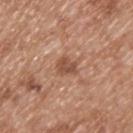notes: no biopsy performed (imaged during a skin exam)
lesion size: ≈2.5 mm
image-analysis metrics: an outline eccentricity of about 0.55 (0 = round, 1 = elongated) and a symmetry-axis asymmetry near 0.25; about 10 CIELAB-L* units darker than the surrounding skin
imaging modality: 15 mm crop, total-body photography
body site: the back
patient: male, approximately 65 years of age